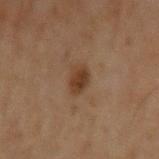The lesion was tiled from a total-body skin photograph and was not biopsied.
Automated image analysis of the tile measured a footprint of about 5 mm², an eccentricity of roughly 0.5, and a shape-asymmetry score of about 0.25 (0 = symmetric). And it measured a lesion color around L≈28 a*≈14 b*≈24 in CIELAB, about 8 CIELAB-L* units darker than the surrounding skin, and a normalized border contrast of about 8.5.
Located on the arm.
The patient is a male aged 68 to 72.
A lesion tile, about 15 mm wide, cut from a 3D total-body photograph.
The tile uses cross-polarized illumination.